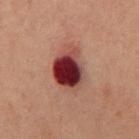lesion size: ≈5 mm | image source: 15 mm crop, total-body photography | location: the chest | subject: male, aged 58–62 | automated lesion analysis: an area of roughly 14 mm² and an outline eccentricity of about 0.75 (0 = round, 1 = elongated); a lesion color around L≈27 a*≈24 b*≈18 in CIELAB and about 17 CIELAB-L* units darker than the surrounding skin; a border-irregularity index near 2.5/10, a within-lesion color-variation index near 8.5/10, and a peripheral color-asymmetry measure near 2.